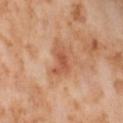biopsy status: no biopsy performed (imaged during a skin exam); imaging modality: ~15 mm crop, total-body skin-cancer survey; anatomic site: the abdomen; patient: female, in their mid- to late 50s; illumination: cross-polarized.